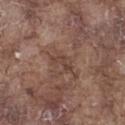{
  "biopsy_status": "not biopsied; imaged during a skin examination",
  "lesion_size": {
    "long_diameter_mm_approx": 3.0
  },
  "patient": {
    "sex": "male",
    "age_approx": 75
  },
  "image": {
    "source": "total-body photography crop",
    "field_of_view_mm": 15
  },
  "lighting": "white-light",
  "automated_metrics": {
    "cielab_L": 41,
    "cielab_a": 19,
    "cielab_b": 24,
    "vs_skin_darker_L": 7.0,
    "vs_skin_contrast_norm": 6.0,
    "nevus_likeness_0_100": 0,
    "lesion_detection_confidence_0_100": 60
  },
  "site": "leg"
}